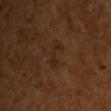A 15 mm close-up extracted from a 3D total-body photography capture. The lesion's longest dimension is about 3 mm. The lesion-visualizer software estimated a border-irregularity index near 8/10, a within-lesion color-variation index near 0/10, and radial color variation of about 0. A female subject aged 53 to 57. Imaged with cross-polarized lighting. From the front of the torso.